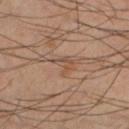Q: Was a biopsy performed?
A: imaged on a skin check; not biopsied
Q: Lesion location?
A: the leg
Q: What kind of image is this?
A: 15 mm crop, total-body photography
Q: How was the tile lit?
A: cross-polarized
Q: How large is the lesion?
A: ~2.5 mm (longest diameter)
Q: Patient demographics?
A: male, roughly 40 years of age